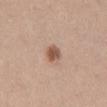{
  "biopsy_status": "not biopsied; imaged during a skin examination",
  "lighting": "white-light",
  "image": {
    "source": "total-body photography crop",
    "field_of_view_mm": 15
  },
  "patient": {
    "sex": "male",
    "age_approx": 35
  },
  "automated_metrics": {
    "area_mm2_approx": 4.0,
    "eccentricity": 0.65,
    "shape_asymmetry": 0.25,
    "cielab_L": 54,
    "cielab_a": 19,
    "cielab_b": 29,
    "vs_skin_contrast_norm": 9.0
  },
  "site": "lower back",
  "lesion_size": {
    "long_diameter_mm_approx": 2.5
  }
}